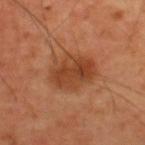The lesion is located on the back.
The recorded lesion diameter is about 5 mm.
A region of skin cropped from a whole-body photographic capture, roughly 15 mm wide.
A male subject, in their 60s.